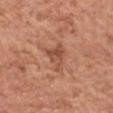Captured during whole-body skin photography for melanoma surveillance; the lesion was not biopsied.
Approximately 4 mm at its widest.
The subject is a male about 70 years old.
A 15 mm close-up tile from a total-body photography series done for melanoma screening.
On the head or neck.
Captured under white-light illumination.